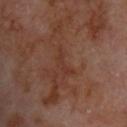The lesion was photographed on a routine skin check and not biopsied; there is no pathology result. The lesion's longest dimension is about 3.5 mm. A roughly 15 mm field-of-view crop from a total-body skin photograph. The lesion is on the upper back. The tile uses cross-polarized illumination. A male patient aged around 70.